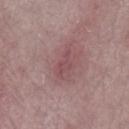workup = catalogued during a skin exam; not biopsied
imaging modality = ~15 mm crop, total-body skin-cancer survey
tile lighting = white-light
subject = female, aged 58 to 62
lesion size = ≈3.5 mm
site = the leg